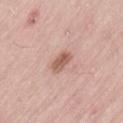Assessment:
Part of a total-body skin-imaging series; this lesion was reviewed on a skin check and was not flagged for biopsy.
Context:
A 15 mm crop from a total-body photograph taken for skin-cancer surveillance. The lesion is on the left thigh. A male subject, approximately 55 years of age.